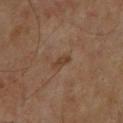The total-body-photography lesion software estimated a lesion area of about 2 mm² and two-axis asymmetry of about 0.3. And it measured an average lesion color of about L≈35 a*≈17 b*≈27 (CIELAB), roughly 7 lightness units darker than nearby skin, and a normalized lesion–skin contrast near 7. The analysis additionally found a border-irregularity rating of about 3/10, a color-variation rating of about 0/10, and radial color variation of about 0. The analysis additionally found a nevus-likeness score of about 0/100 and a detector confidence of about 100 out of 100 that the crop contains a lesion. About 2.5 mm across. Located on the chest. Captured under cross-polarized illumination. Cropped from a total-body skin-imaging series; the visible field is about 15 mm. A male subject aged around 60.